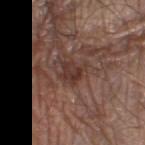Case summary:
- notes — no biopsy performed (imaged during a skin exam)
- lighting — white-light
- body site — the left thigh
- image — ~15 mm crop, total-body skin-cancer survey
- patient — male, roughly 70 years of age
- size — ~3 mm (longest diameter)
- automated metrics — an average lesion color of about L≈35 a*≈19 b*≈23 (CIELAB) and a normalized lesion–skin contrast near 7.5; border irregularity of about 3.5 on a 0–10 scale and a peripheral color-asymmetry measure near 1